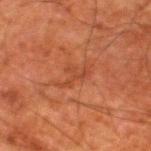The lesion was photographed on a routine skin check and not biopsied; there is no pathology result. Captured under cross-polarized illumination. Located on the left lower leg. A 15 mm close-up extracted from a 3D total-body photography capture. Automated image analysis of the tile measured an automated nevus-likeness rating near 0 out of 100 and lesion-presence confidence of about 95/100. The patient is a male aged 78 to 82.